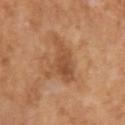workup: imaged on a skin check; not biopsied
lesion size: about 5 mm
image source: ~15 mm tile from a whole-body skin photo
body site: the left upper arm
subject: male, about 75 years old
TBP lesion metrics: an area of roughly 11 mm² and a shape-asymmetry score of about 0.45 (0 = symmetric); an average lesion color of about L≈51 a*≈23 b*≈35 (CIELAB), roughly 9 lightness units darker than nearby skin, and a lesion-to-skin contrast of about 6.5 (normalized; higher = more distinct); a border-irregularity rating of about 6.5/10, a within-lesion color-variation index near 4/10, and peripheral color asymmetry of about 1.5; a nevus-likeness score of about 0/100 and lesion-presence confidence of about 100/100